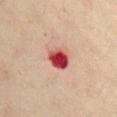• workup: catalogued during a skin exam; not biopsied
• diameter: about 3 mm
• lighting: cross-polarized illumination
• location: the chest
• patient: female, aged 58 to 62
• imaging modality: ~15 mm crop, total-body skin-cancer survey